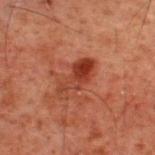Clinical impression:
Part of a total-body skin-imaging series; this lesion was reviewed on a skin check and was not flagged for biopsy.
Acquisition and patient details:
A male subject, aged 58 to 62. The lesion's longest dimension is about 5 mm. The tile uses cross-polarized illumination. Cropped from a whole-body photographic skin survey; the tile spans about 15 mm. The lesion is located on the upper back.